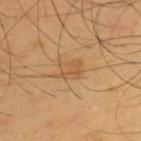illumination: cross-polarized | imaging modality: ~15 mm crop, total-body skin-cancer survey | location: the upper back | subject: male, about 55 years old | automated metrics: an average lesion color of about L≈53 a*≈19 b*≈37 (CIELAB) and a lesion-to-skin contrast of about 5 (normalized; higher = more distinct) | diameter: ~3.5 mm (longest diameter).